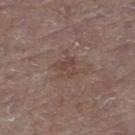Case summary:
* notes — no biopsy performed (imaged during a skin exam)
* subject — female, aged 83 or over
* tile lighting — white-light
* site — the left thigh
* size — ~2.5 mm (longest diameter)
* acquisition — total-body-photography crop, ~15 mm field of view